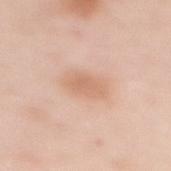Impression:
Recorded during total-body skin imaging; not selected for excision or biopsy.
Acquisition and patient details:
Longest diameter approximately 4 mm. The lesion is on the mid back. A female patient, approximately 50 years of age. A close-up tile cropped from a whole-body skin photograph, about 15 mm across.